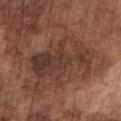The lesion was tiled from a total-body skin photograph and was not biopsied. A close-up tile cropped from a whole-body skin photograph, about 15 mm across. This is a white-light tile. From the chest. A male subject, aged 73–77.